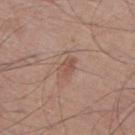Part of a total-body skin-imaging series; this lesion was reviewed on a skin check and was not flagged for biopsy.
A 15 mm close-up extracted from a 3D total-body photography capture.
On the left thigh.
A male patient, aged 73–77.
Imaged with white-light lighting.
The lesion-visualizer software estimated an area of roughly 3.5 mm² and a shape-asymmetry score of about 0.3 (0 = symmetric). It also reported a lesion color around L≈52 a*≈19 b*≈26 in CIELAB. And it measured border irregularity of about 2.5 on a 0–10 scale and radial color variation of about 0.5. The analysis additionally found lesion-presence confidence of about 100/100.